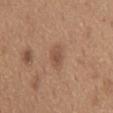A roughly 15 mm field-of-view crop from a total-body skin photograph.
Imaged with white-light lighting.
The lesion is on the mid back.
The subject is a male in their mid-60s.
Automated tile analysis of the lesion measured an area of roughly 4.5 mm² and two-axis asymmetry of about 0.15. And it measured a mean CIELAB color near L≈51 a*≈19 b*≈29 and about 8 CIELAB-L* units darker than the surrounding skin.
Measured at roughly 3 mm in maximum diameter.